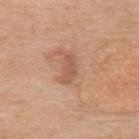  biopsy_status: not biopsied; imaged during a skin examination
  lighting: white-light
  automated_metrics:
    area_mm2_approx: 5.0
    eccentricity: 0.85
    shape_asymmetry: 0.35
    lesion_detection_confidence_0_100: 100
  lesion_size:
    long_diameter_mm_approx: 3.5
  image:
    source: total-body photography crop
    field_of_view_mm: 15
  patient:
    sex: female
    age_approx: 75
  site: back